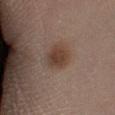The lesion was photographed on a routine skin check and not biopsied; there is no pathology result.